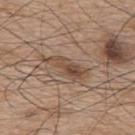Q: Was this lesion biopsied?
A: imaged on a skin check; not biopsied
Q: Lesion size?
A: about 5.5 mm
Q: Automated lesion metrics?
A: a footprint of about 8.5 mm², a shape eccentricity near 0.95, and a shape-asymmetry score of about 0.4 (0 = symmetric); a mean CIELAB color near L≈48 a*≈16 b*≈28, about 10 CIELAB-L* units darker than the surrounding skin, and a normalized lesion–skin contrast near 7.5; a border-irregularity index near 5/10 and peripheral color asymmetry of about 2.5; a nevus-likeness score of about 45/100 and a lesion-detection confidence of about 100/100
Q: Patient demographics?
A: male, aged 73 to 77
Q: What is the imaging modality?
A: ~15 mm tile from a whole-body skin photo
Q: What is the anatomic site?
A: the upper back